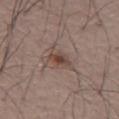Imaged during a routine full-body skin examination; the lesion was not biopsied and no histopathology is available. The recorded lesion diameter is about 3 mm. The total-body-photography lesion software estimated roughly 10 lightness units darker than nearby skin and a normalized lesion–skin contrast near 8.5. The analysis additionally found a classifier nevus-likeness of about 85/100 and lesion-presence confidence of about 100/100. The lesion is on the abdomen. A lesion tile, about 15 mm wide, cut from a 3D total-body photograph. A male patient, roughly 75 years of age. This is a white-light tile.